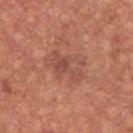subject — male, aged around 65; location — the chest; lesion diameter — about 5 mm; imaging modality — total-body-photography crop, ~15 mm field of view; lighting — white-light.